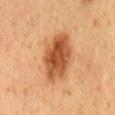Findings:
* automated lesion analysis — a lesion–skin lightness drop of about 13 and a normalized lesion–skin contrast near 9.5
* subject — male, aged around 60
* image source — ~15 mm crop, total-body skin-cancer survey
* lesion size — about 7 mm
* location — the back